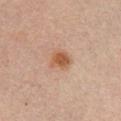Acquisition and patient details: The lesion is on the chest. Imaged with cross-polarized lighting. The patient is a male aged approximately 30. The lesion-visualizer software estimated a lesion color around L≈45 a*≈18 b*≈28 in CIELAB, about 9 CIELAB-L* units darker than the surrounding skin, and a lesion-to-skin contrast of about 8 (normalized; higher = more distinct). It also reported a border-irregularity index near 3/10 and internal color variation of about 3 on a 0–10 scale. A close-up tile cropped from a whole-body skin photograph, about 15 mm across.